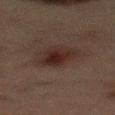The lesion was tiled from a total-body skin photograph and was not biopsied.
The tile uses cross-polarized illumination.
The lesion is located on the mid back.
A region of skin cropped from a whole-body photographic capture, roughly 15 mm wide.
About 4 mm across.
The patient is a male aged approximately 50.